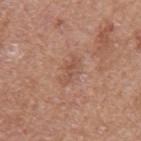image: total-body-photography crop, ~15 mm field of view
size: ≈3.5 mm
patient: female, roughly 55 years of age
image-analysis metrics: an area of roughly 4 mm² and a shape eccentricity near 0.9; a lesion–skin lightness drop of about 7 and a lesion-to-skin contrast of about 5 (normalized; higher = more distinct); a nevus-likeness score of about 0/100 and a lesion-detection confidence of about 100/100
anatomic site: the left upper arm